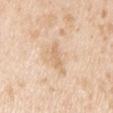biopsy status — no biopsy performed (imaged during a skin exam) | illumination — white-light illumination | subject — male, aged approximately 45 | size — ≈2.5 mm | anatomic site — the left upper arm | TBP lesion metrics — a lesion area of about 2.5 mm² and a symmetry-axis asymmetry near 0.45; a border-irregularity index near 5/10, internal color variation of about 0 on a 0–10 scale, and a peripheral color-asymmetry measure near 0; a lesion-detection confidence of about 100/100 | acquisition — ~15 mm crop, total-body skin-cancer survey.